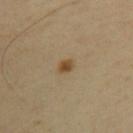The lesion was photographed on a routine skin check and not biopsied; there is no pathology result. The lesion is on the upper back. A male subject about 55 years old. Measured at roughly 2 mm in maximum diameter. A 15 mm crop from a total-body photograph taken for skin-cancer surveillance. Imaged with cross-polarized lighting. The total-body-photography lesion software estimated a lesion area of about 3 mm² and two-axis asymmetry of about 0.2. And it measured an average lesion color of about L≈47 a*≈14 b*≈35 (CIELAB) and a lesion–skin lightness drop of about 10. And it measured an automated nevus-likeness rating near 95 out of 100 and a detector confidence of about 100 out of 100 that the crop contains a lesion.